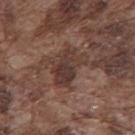Q: Was this lesion biopsied?
A: catalogued during a skin exam; not biopsied
Q: What kind of image is this?
A: ~15 mm crop, total-body skin-cancer survey
Q: Lesion location?
A: the mid back
Q: Patient demographics?
A: male, aged 73–77
Q: How was the tile lit?
A: white-light
Q: What is the lesion's diameter?
A: about 4.5 mm
Q: What did automated image analysis measure?
A: a border-irregularity index near 4.5/10, a color-variation rating of about 4.5/10, and radial color variation of about 1.5; an automated nevus-likeness rating near 0 out of 100 and a lesion-detection confidence of about 95/100